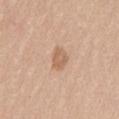Impression: This lesion was catalogued during total-body skin photography and was not selected for biopsy. Clinical summary: This image is a 15 mm lesion crop taken from a total-body photograph. A female subject approximately 25 years of age. From the mid back.